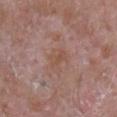Case summary:
- biopsy status — total-body-photography surveillance lesion; no biopsy